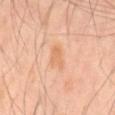Impression:
Part of a total-body skin-imaging series; this lesion was reviewed on a skin check and was not flagged for biopsy.